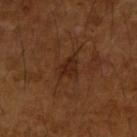Notes:
* workup · no biopsy performed (imaged during a skin exam)
* image · ~15 mm crop, total-body skin-cancer survey
* tile lighting · cross-polarized illumination
* location · the right upper arm
* subject · male, aged approximately 65
* lesion size · about 2.5 mm
* automated metrics · a border-irregularity rating of about 3.5/10, a color-variation rating of about 1.5/10, and peripheral color asymmetry of about 0.5